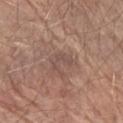Q: Was a biopsy performed?
A: total-body-photography surveillance lesion; no biopsy
Q: What kind of image is this?
A: ~15 mm tile from a whole-body skin photo
Q: What is the anatomic site?
A: the left forearm
Q: What are the patient's age and sex?
A: male, approximately 70 years of age